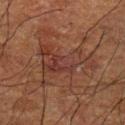A male subject aged around 60. On the left forearm. Cropped from a total-body skin-imaging series; the visible field is about 15 mm. Measured at roughly 7.5 mm in maximum diameter.